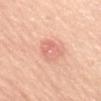follow-up = no biopsy performed (imaged during a skin exam) | lighting = white-light | image-analysis metrics = a shape eccentricity near 0.7 and a symmetry-axis asymmetry near 0.2; an average lesion color of about L≈69 a*≈27 b*≈30 (CIELAB), about 8 CIELAB-L* units darker than the surrounding skin, and a normalized border contrast of about 5; a border-irregularity index near 2/10, a within-lesion color-variation index near 3.5/10, and radial color variation of about 1.5; a nevus-likeness score of about 15/100 and a detector confidence of about 100 out of 100 that the crop contains a lesion | diameter = about 3.5 mm | subject = female, about 70 years old | body site = the mid back | imaging modality = total-body-photography crop, ~15 mm field of view.